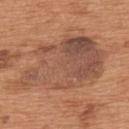biopsy_status: not biopsied; imaged during a skin examination
lighting: white-light
site: upper back
patient:
  sex: male
  age_approx: 65
image:
  source: total-body photography crop
  field_of_view_mm: 15
lesion_size:
  long_diameter_mm_approx: 12.0